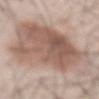Clinical summary: The patient is a male in their mid-50s. An algorithmic analysis of the crop reported a shape-asymmetry score of about 0.3 (0 = symmetric). It also reported an average lesion color of about L≈58 a*≈16 b*≈24 (CIELAB), roughly 11 lightness units darker than nearby skin, and a normalized border contrast of about 7.5. And it measured a border-irregularity index near 4.5/10, internal color variation of about 7.5 on a 0–10 scale, and a peripheral color-asymmetry measure near 3. And it measured a nevus-likeness score of about 100/100. The tile uses white-light illumination. From the abdomen. This image is a 15 mm lesion crop taken from a total-body photograph. Longest diameter approximately 13 mm.